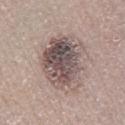- follow-up · total-body-photography surveillance lesion; no biopsy
- illumination · white-light
- subject · male, aged approximately 65
- acquisition · total-body-photography crop, ~15 mm field of view
- site · the right lower leg
- lesion diameter · about 6.5 mm